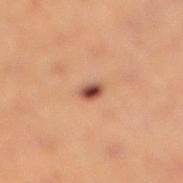Assessment:
Part of a total-body skin-imaging series; this lesion was reviewed on a skin check and was not flagged for biopsy.
Image and clinical context:
Measured at roughly 2.5 mm in maximum diameter. A roughly 15 mm field-of-view crop from a total-body skin photograph. Imaged with cross-polarized lighting. A female patient aged 33–37. The lesion is on the right lower leg. Automated tile analysis of the lesion measured a lesion area of about 3 mm², an eccentricity of roughly 0.8, and two-axis asymmetry of about 0.25. And it measured a nevus-likeness score of about 100/100 and a detector confidence of about 100 out of 100 that the crop contains a lesion.